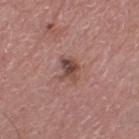The lesion was tiled from a total-body skin photograph and was not biopsied. This image is a 15 mm lesion crop taken from a total-body photograph. Located on the right lower leg. The subject is a male approximately 75 years of age. Longest diameter approximately 2.5 mm.